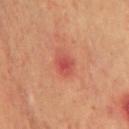The lesion was tiled from a total-body skin photograph and was not biopsied. Captured under cross-polarized illumination. The total-body-photography lesion software estimated roughly 9 lightness units darker than nearby skin and a normalized border contrast of about 6.5. A 15 mm crop from a total-body photograph taken for skin-cancer surveillance. The lesion is on the chest. A male subject aged approximately 70. About 2.5 mm across.